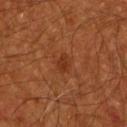Notes:
• follow-up — no biopsy performed (imaged during a skin exam)
• site — the upper back
• image source — 15 mm crop, total-body photography
• patient — male, about 60 years old
• tile lighting — cross-polarized illumination